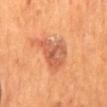biopsy status: total-body-photography surveillance lesion; no biopsy | lighting: cross-polarized illumination | image source: 15 mm crop, total-body photography | body site: the mid back | subject: male, approximately 55 years of age.